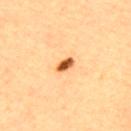Assessment:
This lesion was catalogued during total-body skin photography and was not selected for biopsy.
Background:
On the upper back. A male patient roughly 60 years of age. Cropped from a whole-body photographic skin survey; the tile spans about 15 mm.